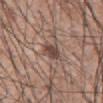Clinical impression: The lesion was tiled from a total-body skin photograph and was not biopsied. Acquisition and patient details: A 15 mm close-up extracted from a 3D total-body photography capture. The lesion-visualizer software estimated an average lesion color of about L≈46 a*≈17 b*≈22 (CIELAB), a lesion–skin lightness drop of about 12, and a normalized lesion–skin contrast near 9. The analysis additionally found border irregularity of about 2.5 on a 0–10 scale and a peripheral color-asymmetry measure near 0.5. It also reported a nevus-likeness score of about 35/100 and lesion-presence confidence of about 100/100. Captured under white-light illumination. Located on the abdomen. A male subject aged around 60.